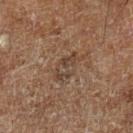Impression: The lesion was photographed on a routine skin check and not biopsied; there is no pathology result. Acquisition and patient details: This image is a 15 mm lesion crop taken from a total-body photograph. Located on the leg. This is a cross-polarized tile. A male patient approximately 60 years of age.